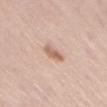| field | value |
|---|---|
| tile lighting | white-light illumination |
| imaging modality | ~15 mm crop, total-body skin-cancer survey |
| location | the left thigh |
| automated metrics | an area of roughly 4 mm², an eccentricity of roughly 0.85, and a symmetry-axis asymmetry near 0.2; a border-irregularity index near 2.5/10, a within-lesion color-variation index near 2.5/10, and peripheral color asymmetry of about 1 |
| subject | male, approximately 60 years of age |